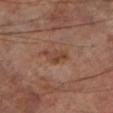Q: Was this lesion biopsied?
A: imaged on a skin check; not biopsied
Q: Automated lesion metrics?
A: a border-irregularity rating of about 4/10, internal color variation of about 3.5 on a 0–10 scale, and a peripheral color-asymmetry measure near 1; a classifier nevus-likeness of about 0/100 and a lesion-detection confidence of about 100/100
Q: Where on the body is the lesion?
A: the left lower leg
Q: Illumination type?
A: cross-polarized
Q: How large is the lesion?
A: ≈3.5 mm
Q: What is the imaging modality?
A: total-body-photography crop, ~15 mm field of view
Q: Who is the patient?
A: male, aged 68–72